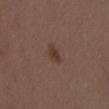No biopsy was performed on this lesion — it was imaged during a full skin examination and was not determined to be concerning. Measured at roughly 2.5 mm in maximum diameter. A region of skin cropped from a whole-body photographic capture, roughly 15 mm wide. Located on the lower back. Captured under white-light illumination. The lesion-visualizer software estimated an eccentricity of roughly 0.65 and a shape-asymmetry score of about 0.3 (0 = symmetric). And it measured an average lesion color of about L≈37 a*≈18 b*≈23 (CIELAB) and roughly 7 lightness units darker than nearby skin. It also reported an automated nevus-likeness rating near 90 out of 100 and a detector confidence of about 100 out of 100 that the crop contains a lesion. A male subject approximately 30 years of age.